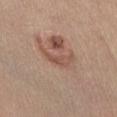Clinical impression:
Part of a total-body skin-imaging series; this lesion was reviewed on a skin check and was not flagged for biopsy.
Background:
The lesion's longest dimension is about 4 mm. Cropped from a total-body skin-imaging series; the visible field is about 15 mm. A female patient in their mid-30s. Located on the abdomen.